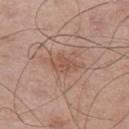Q: How was the tile lit?
A: white-light
Q: Patient demographics?
A: male, aged around 65
Q: What is the anatomic site?
A: the left upper arm
Q: What kind of image is this?
A: ~15 mm crop, total-body skin-cancer survey